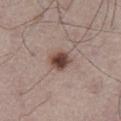biopsy status: imaged on a skin check; not biopsied
lesion size: about 3.5 mm
site: the leg
patient: male, aged 73–77
illumination: white-light illumination
acquisition: 15 mm crop, total-body photography